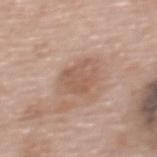site: the upper back | subject: female, approximately 60 years of age | automated lesion analysis: an eccentricity of roughly 0.55 and a symmetry-axis asymmetry near 0.4; a nevus-likeness score of about 0/100 and a detector confidence of about 100 out of 100 that the crop contains a lesion | size: ≈3.5 mm | illumination: white-light illumination | image: 15 mm crop, total-body photography.